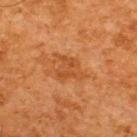Part of a total-body skin-imaging series; this lesion was reviewed on a skin check and was not flagged for biopsy. Longest diameter approximately 4 mm. The lesion is on the upper back. The patient is a male aged around 65. Cropped from a total-body skin-imaging series; the visible field is about 15 mm. Captured under cross-polarized illumination.